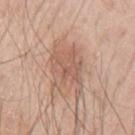site: the arm; diameter: ~6 mm (longest diameter); imaging modality: ~15 mm crop, total-body skin-cancer survey; patient: male, about 60 years old.